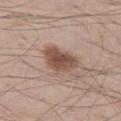workup: no biopsy performed (imaged during a skin exam)
TBP lesion metrics: a lesion area of about 11 mm² and a symmetry-axis asymmetry near 0.35; a border-irregularity rating of about 3/10, internal color variation of about 4 on a 0–10 scale, and radial color variation of about 1
location: the left thigh
illumination: white-light illumination
lesion size: ≈4.5 mm
patient: male, aged 48 to 52
imaging modality: total-body-photography crop, ~15 mm field of view